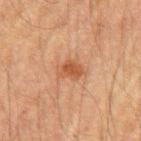The lesion was tiled from a total-body skin photograph and was not biopsied.
A lesion tile, about 15 mm wide, cut from a 3D total-body photograph.
About 3 mm across.
This is a cross-polarized tile.
On the left upper arm.
A male patient, aged approximately 65.